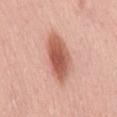• workup: no biopsy performed (imaged during a skin exam)
• illumination: white-light
• lesion size: ~6.5 mm (longest diameter)
• patient: male, aged 53 to 57
• image-analysis metrics: an automated nevus-likeness rating near 100 out of 100 and a detector confidence of about 100 out of 100 that the crop contains a lesion
• location: the mid back
• imaging modality: ~15 mm crop, total-body skin-cancer survey